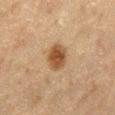– workup — no biopsy performed (imaged during a skin exam)
– subject — male, roughly 75 years of age
– tile lighting — cross-polarized
– body site — the abdomen
– image — 15 mm crop, total-body photography
– size — about 3.5 mm